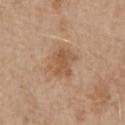Q: Was this lesion biopsied?
A: total-body-photography surveillance lesion; no biopsy
Q: Automated lesion metrics?
A: an automated nevus-likeness rating near 20 out of 100 and a detector confidence of about 100 out of 100 that the crop contains a lesion
Q: Who is the patient?
A: male, about 65 years old
Q: What kind of image is this?
A: total-body-photography crop, ~15 mm field of view
Q: Lesion location?
A: the chest
Q: What lighting was used for the tile?
A: white-light illumination
Q: How large is the lesion?
A: about 3.5 mm